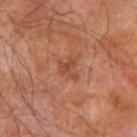{"biopsy_status": "not biopsied; imaged during a skin examination", "image": {"source": "total-body photography crop", "field_of_view_mm": 15}, "automated_metrics": {"area_mm2_approx": 3.5, "eccentricity": 0.6, "cielab_L": 46, "cielab_a": 25, "cielab_b": 33, "vs_skin_darker_L": 8.0, "vs_skin_contrast_norm": 6.0, "border_irregularity_0_10": 7.5, "color_variation_0_10": 0.0, "peripheral_color_asymmetry": 0.0}, "lesion_size": {"long_diameter_mm_approx": 2.5}, "patient": {"sex": "male", "age_approx": 70}, "site": "right forearm", "lighting": "cross-polarized"}